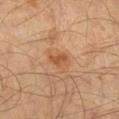follow-up = total-body-photography surveillance lesion; no biopsy
lesion diameter = ~2.5 mm (longest diameter)
acquisition = ~15 mm crop, total-body skin-cancer survey
location = the right lower leg
lighting = cross-polarized
automated lesion analysis = a footprint of about 3.5 mm², a shape eccentricity near 0.8, and a symmetry-axis asymmetry near 0.35; an average lesion color of about L≈54 a*≈25 b*≈38 (CIELAB) and roughly 8 lightness units darker than nearby skin; a nevus-likeness score of about 25/100 and a lesion-detection confidence of about 100/100
subject = male, roughly 40 years of age